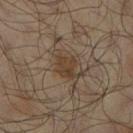| feature | finding |
|---|---|
| workup | imaged on a skin check; not biopsied |
| patient | male, roughly 65 years of age |
| image | 15 mm crop, total-body photography |
| body site | the right lower leg |